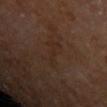The lesion was tiled from a total-body skin photograph and was not biopsied. Automated tile analysis of the lesion measured an area of roughly 4.5 mm² and two-axis asymmetry of about 0.6. And it measured a mean CIELAB color near L≈23 a*≈15 b*≈21, a lesion–skin lightness drop of about 4, and a lesion-to-skin contrast of about 5 (normalized; higher = more distinct). The analysis additionally found a border-irregularity index near 7.5/10 and a peripheral color-asymmetry measure near 0. It also reported a nevus-likeness score of about 0/100 and lesion-presence confidence of about 95/100. Approximately 4.5 mm at its widest. A 15 mm close-up extracted from a 3D total-body photography capture. On the left upper arm. The tile uses cross-polarized illumination. A male subject, aged 63 to 67.